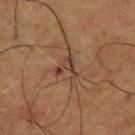Impression: No biopsy was performed on this lesion — it was imaged during a full skin examination and was not determined to be concerning. Clinical summary: Located on the upper back. Captured under cross-polarized illumination. Automated tile analysis of the lesion measured a within-lesion color-variation index near 1.5/10 and radial color variation of about 0.5. It also reported a classifier nevus-likeness of about 0/100 and lesion-presence confidence of about 55/100. The subject is a male roughly 60 years of age. Cropped from a whole-body photographic skin survey; the tile spans about 15 mm.